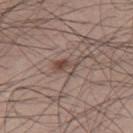No biopsy was performed on this lesion — it was imaged during a full skin examination and was not determined to be concerning. The lesion is located on the right thigh. A male subject, aged around 30. This image is a 15 mm lesion crop taken from a total-body photograph.